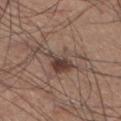- notes — imaged on a skin check; not biopsied
- tile lighting — white-light illumination
- patient — male, approximately 35 years of age
- acquisition — ~15 mm tile from a whole-body skin photo
- anatomic site — the left lower leg
- lesion size — ~9.5 mm (longest diameter)
- TBP lesion metrics — an eccentricity of roughly 0.95 and a shape-asymmetry score of about 0.55 (0 = symmetric); a color-variation rating of about 8/10 and radial color variation of about 2